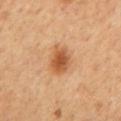This lesion was catalogued during total-body skin photography and was not selected for biopsy. This image is a 15 mm lesion crop taken from a total-body photograph. About 3.5 mm across. From the chest. A male patient, roughly 60 years of age.